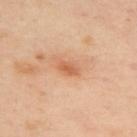| field | value |
|---|---|
| follow-up | catalogued during a skin exam; not biopsied |
| image source | total-body-photography crop, ~15 mm field of view |
| body site | the upper back |
| patient | female, roughly 40 years of age |